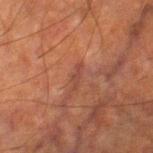| feature | finding |
|---|---|
| notes | imaged on a skin check; not biopsied |
| automated metrics | a lesion area of about 2 mm², a shape eccentricity near 0.95, and a symmetry-axis asymmetry near 0.5; a lesion color around L≈35 a*≈21 b*≈24 in CIELAB, roughly 6 lightness units darker than nearby skin, and a normalized lesion–skin contrast near 5.5; a border-irregularity rating of about 6/10 and peripheral color asymmetry of about 0 |
| illumination | cross-polarized illumination |
| body site | the leg |
| image source | ~15 mm tile from a whole-body skin photo |
| patient | male, about 65 years old |
| size | about 2.5 mm |